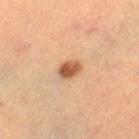Assessment: This lesion was catalogued during total-body skin photography and was not selected for biopsy. Context: Imaged with cross-polarized lighting. The total-body-photography lesion software estimated an area of roughly 4.5 mm², an outline eccentricity of about 0.65 (0 = round, 1 = elongated), and a shape-asymmetry score of about 0.2 (0 = symmetric). The analysis additionally found a classifier nevus-likeness of about 95/100 and a lesion-detection confidence of about 100/100. Located on the right thigh. A female subject, aged 53 to 57. A roughly 15 mm field-of-view crop from a total-body skin photograph.